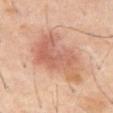Q: Was this lesion biopsied?
A: catalogued during a skin exam; not biopsied
Q: What is the imaging modality?
A: ~15 mm tile from a whole-body skin photo
Q: How large is the lesion?
A: about 7 mm
Q: What are the patient's age and sex?
A: male, aged around 60
Q: What is the anatomic site?
A: the front of the torso
Q: What did automated image analysis measure?
A: a lesion area of about 23 mm², an outline eccentricity of about 0.75 (0 = round, 1 = elongated), and two-axis asymmetry of about 0.35; lesion-presence confidence of about 100/100